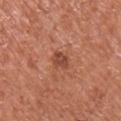- notes · imaged on a skin check; not biopsied
- anatomic site · the arm
- imaging modality · ~15 mm tile from a whole-body skin photo
- lighting · white-light
- subject · male, roughly 65 years of age